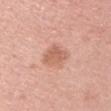Findings:
– biopsy status: imaged on a skin check; not biopsied